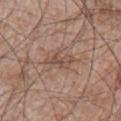This lesion was catalogued during total-body skin photography and was not selected for biopsy.
The subject is a male approximately 65 years of age.
From the left upper arm.
An algorithmic analysis of the crop reported border irregularity of about 6 on a 0–10 scale and peripheral color asymmetry of about 0.5.
A 15 mm crop from a total-body photograph taken for skin-cancer surveillance.
The tile uses white-light illumination.
The recorded lesion diameter is about 3.5 mm.